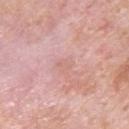Imaged during a routine full-body skin examination; the lesion was not biopsied and no histopathology is available. The lesion is on the upper back. A male subject, aged 53 to 57. Measured at roughly 2 mm in maximum diameter. An algorithmic analysis of the crop reported about 4 CIELAB-L* units darker than the surrounding skin and a normalized lesion–skin contrast near 4. And it measured a border-irregularity index near 3/10, a color-variation rating of about 0/10, and a peripheral color-asymmetry measure near 0. A roughly 15 mm field-of-view crop from a total-body skin photograph.